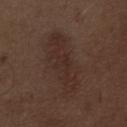workup: no biopsy performed (imaged during a skin exam)
automated lesion analysis: a border-irregularity rating of about 4/10, a color-variation rating of about 3/10, and peripheral color asymmetry of about 1; an automated nevus-likeness rating near 30 out of 100 and a detector confidence of about 100 out of 100 that the crop contains a lesion
subject: male, aged 68 to 72
tile lighting: white-light
anatomic site: the front of the torso
image source: ~15 mm tile from a whole-body skin photo
diameter: ≈8 mm